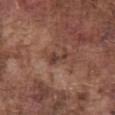{
  "biopsy_status": "not biopsied; imaged during a skin examination",
  "lighting": "white-light",
  "image": {
    "source": "total-body photography crop",
    "field_of_view_mm": 15
  },
  "patient": {
    "sex": "male",
    "age_approx": 75
  },
  "site": "abdomen",
  "lesion_size": {
    "long_diameter_mm_approx": 2.5
  }
}